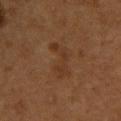| key | value |
|---|---|
| imaging modality | ~15 mm crop, total-body skin-cancer survey |
| site | the upper back |
| size | ≈5 mm |
| patient | male, aged approximately 55 |